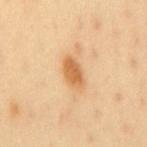Assessment:
The lesion was tiled from a total-body skin photograph and was not biopsied.
Clinical summary:
This image is a 15 mm lesion crop taken from a total-body photograph. An algorithmic analysis of the crop reported an outline eccentricity of about 0.85 (0 = round, 1 = elongated) and two-axis asymmetry of about 0.2. The software also gave a classifier nevus-likeness of about 95/100. A male subject, aged 38 to 42. The lesion is located on the mid back. Measured at roughly 4 mm in maximum diameter. The tile uses cross-polarized illumination.